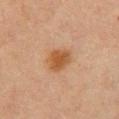follow-up: catalogued during a skin exam; not biopsied
subject: female, in their mid-60s
illumination: cross-polarized illumination
image: total-body-photography crop, ~15 mm field of view
TBP lesion metrics: an outline eccentricity of about 0.6 (0 = round, 1 = elongated) and a shape-asymmetry score of about 0.15 (0 = symmetric); an average lesion color of about L≈46 a*≈20 b*≈34 (CIELAB) and roughly 9 lightness units darker than nearby skin; a detector confidence of about 100 out of 100 that the crop contains a lesion
site: the chest
size: ≈3.5 mm